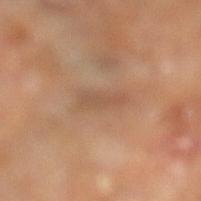The lesion was photographed on a routine skin check and not biopsied; there is no pathology result. The patient is a male aged 68–72. On the left lower leg. Cropped from a whole-body photographic skin survey; the tile spans about 15 mm.